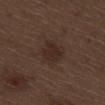This lesion was catalogued during total-body skin photography and was not selected for biopsy. The recorded lesion diameter is about 3 mm. A male patient aged 68–72. The lesion is on the right thigh. Imaged with white-light lighting. A 15 mm crop from a total-body photograph taken for skin-cancer surveillance.